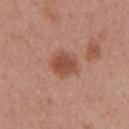Notes:
* workup · no biopsy performed (imaged during a skin exam)
* patient · female, aged approximately 50
* body site · the left upper arm
* imaging modality · ~15 mm crop, total-body skin-cancer survey
* lesion size · about 3.5 mm
* tile lighting · white-light illumination
* automated lesion analysis · a lesion color around L≈50 a*≈24 b*≈30 in CIELAB, about 10 CIELAB-L* units darker than the surrounding skin, and a lesion-to-skin contrast of about 8 (normalized; higher = more distinct); a color-variation rating of about 3/10; a lesion-detection confidence of about 100/100